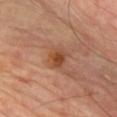Clinical impression: The lesion was photographed on a routine skin check and not biopsied; there is no pathology result. Context: A roughly 15 mm field-of-view crop from a total-body skin photograph. The lesion is on the chest. Measured at roughly 2.5 mm in maximum diameter. Automated tile analysis of the lesion measured a shape eccentricity near 0.55 and two-axis asymmetry of about 0.15. The software also gave a nevus-likeness score of about 60/100. A male patient approximately 65 years of age.